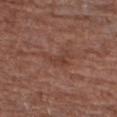Impression: The lesion was photographed on a routine skin check and not biopsied; there is no pathology result. Background: A region of skin cropped from a whole-body photographic capture, roughly 15 mm wide. The lesion's longest dimension is about 3.5 mm. The tile uses white-light illumination. Automated image analysis of the tile measured an area of roughly 5 mm², a shape eccentricity near 0.75, and a shape-asymmetry score of about 0.65 (0 = symmetric). The analysis additionally found an average lesion color of about L≈41 a*≈22 b*≈27 (CIELAB), roughly 6 lightness units darker than nearby skin, and a normalized lesion–skin contrast near 5.5. The software also gave a border-irregularity index near 7.5/10. The software also gave a classifier nevus-likeness of about 0/100 and lesion-presence confidence of about 95/100. On the right forearm. A male patient, roughly 70 years of age.